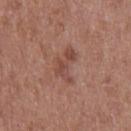Part of a total-body skin-imaging series; this lesion was reviewed on a skin check and was not flagged for biopsy.
Cropped from a total-body skin-imaging series; the visible field is about 15 mm.
Automated image analysis of the tile measured a lesion area of about 6.5 mm² and an eccentricity of roughly 0.85. It also reported an average lesion color of about L≈47 a*≈23 b*≈26 (CIELAB) and a lesion-to-skin contrast of about 6 (normalized; higher = more distinct). And it measured border irregularity of about 8 on a 0–10 scale, a within-lesion color-variation index near 2.5/10, and a peripheral color-asymmetry measure near 1.
On the right thigh.
A female subject in their 40s.